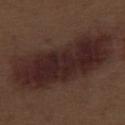notes: total-body-photography surveillance lesion; no biopsy | size: ≈13.5 mm | site: the right thigh | image: total-body-photography crop, ~15 mm field of view | TBP lesion metrics: an eccentricity of roughly 0.95; border irregularity of about 3.5 on a 0–10 scale and a peripheral color-asymmetry measure near 1.5; a nevus-likeness score of about 10/100 and a detector confidence of about 100 out of 100 that the crop contains a lesion | subject: male, aged around 70 | lighting: white-light illumination.